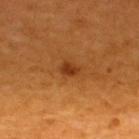Q: Is there a histopathology result?
A: no biopsy performed (imaged during a skin exam)
Q: What is the anatomic site?
A: the upper back
Q: What kind of image is this?
A: total-body-photography crop, ~15 mm field of view
Q: Who is the patient?
A: male, aged around 60